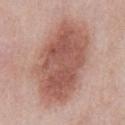Imaged during a routine full-body skin examination; the lesion was not biopsied and no histopathology is available. The total-body-photography lesion software estimated a nevus-likeness score of about 85/100 and a detector confidence of about 100 out of 100 that the crop contains a lesion. The lesion is on the abdomen. The tile uses white-light illumination. Cropped from a whole-body photographic skin survey; the tile spans about 15 mm. A male patient, aged 53–57.